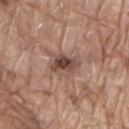This lesion was catalogued during total-body skin photography and was not selected for biopsy. From the lower back. This is a white-light tile. A 15 mm close-up extracted from a 3D total-body photography capture. The patient is a male aged 78–82.